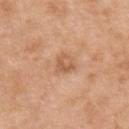{"biopsy_status": "not biopsied; imaged during a skin examination", "patient": {"sex": "male", "age_approx": 50}, "site": "upper back", "image": {"source": "total-body photography crop", "field_of_view_mm": 15}}